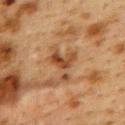No biopsy was performed on this lesion — it was imaged during a full skin examination and was not determined to be concerning. A roughly 15 mm field-of-view crop from a total-body skin photograph. About 5 mm across. A female patient, aged around 40. This is a cross-polarized tile. From the upper back.